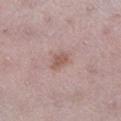Impression:
Captured during whole-body skin photography for melanoma surveillance; the lesion was not biopsied.
Clinical summary:
Located on the right lower leg. A lesion tile, about 15 mm wide, cut from a 3D total-body photograph. A female patient in their mid-40s.